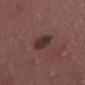Imaged during a routine full-body skin examination; the lesion was not biopsied and no histopathology is available.
Automated image analysis of the tile measured an average lesion color of about L≈31 a*≈17 b*≈16 (CIELAB) and a lesion–skin lightness drop of about 10. The software also gave internal color variation of about 2.5 on a 0–10 scale and peripheral color asymmetry of about 1. The software also gave an automated nevus-likeness rating near 45 out of 100 and a lesion-detection confidence of about 100/100.
A female patient, approximately 40 years of age.
Captured under white-light illumination.
About 3.5 mm across.
The lesion is on the leg.
This image is a 15 mm lesion crop taken from a total-body photograph.